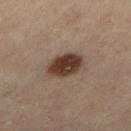Case summary:
• workup: catalogued during a skin exam; not biopsied
• patient: male, about 55 years old
• automated metrics: a footprint of about 11 mm² and two-axis asymmetry of about 0.1; a lesion color around L≈30 a*≈14 b*≈20 in CIELAB, a lesion–skin lightness drop of about 12, and a lesion-to-skin contrast of about 11.5 (normalized; higher = more distinct); a border-irregularity rating of about 1/10, a color-variation rating of about 4/10, and a peripheral color-asymmetry measure near 1; a classifier nevus-likeness of about 100/100 and lesion-presence confidence of about 100/100
• imaging modality: total-body-photography crop, ~15 mm field of view
• anatomic site: the leg
• lighting: cross-polarized illumination
• size: about 4 mm